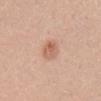{"biopsy_status": "not biopsied; imaged during a skin examination", "image": {"source": "total-body photography crop", "field_of_view_mm": 15}, "site": "abdomen", "automated_metrics": {"area_mm2_approx": 6.0, "eccentricity": 0.85, "shape_asymmetry": 0.25, "border_irregularity_0_10": 2.5, "color_variation_0_10": 5.0}, "patient": {"sex": "female", "age_approx": 35}, "lesion_size": {"long_diameter_mm_approx": 3.5}}